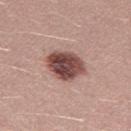The lesion was photographed on a routine skin check and not biopsied; there is no pathology result.
A female subject approximately 25 years of age.
The lesion is on the leg.
A lesion tile, about 15 mm wide, cut from a 3D total-body photograph.
Longest diameter approximately 4.5 mm.
Imaged with white-light lighting.
An algorithmic analysis of the crop reported an eccentricity of roughly 0.6 and two-axis asymmetry of about 0.15. The software also gave a border-irregularity rating of about 1.5/10, a color-variation rating of about 6.5/10, and a peripheral color-asymmetry measure near 2. The analysis additionally found a lesion-detection confidence of about 100/100.